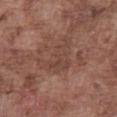notes = total-body-photography surveillance lesion; no biopsy
acquisition = total-body-photography crop, ~15 mm field of view
site = the abdomen
diameter = ≈5.5 mm
subject = male, in their mid- to late 70s